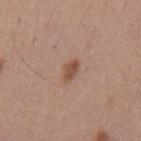Case summary:
– follow-up · catalogued during a skin exam; not biopsied
– location · the mid back
– image · 15 mm crop, total-body photography
– patient · male, approximately 55 years of age